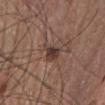Part of a total-body skin-imaging series; this lesion was reviewed on a skin check and was not flagged for biopsy. The lesion is on the chest. About 3 mm across. A 15 mm close-up extracted from a 3D total-body photography capture. This is a white-light tile. The patient is a female aged around 65.